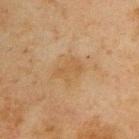{
  "biopsy_status": "not biopsied; imaged during a skin examination"
}